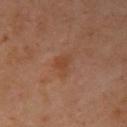Clinical impression: Recorded during total-body skin imaging; not selected for excision or biopsy. Context: The subject is a female about 55 years old. Located on the arm. Longest diameter approximately 3 mm. Automated tile analysis of the lesion measured a lesion color around L≈45 a*≈23 b*≈33 in CIELAB and about 6 CIELAB-L* units darker than the surrounding skin. This image is a 15 mm lesion crop taken from a total-body photograph.